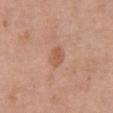The lesion was tiled from a total-body skin photograph and was not biopsied.
Measured at roughly 3 mm in maximum diameter.
The subject is a female about 60 years old.
Cropped from a whole-body photographic skin survey; the tile spans about 15 mm.
Captured under white-light illumination.
The lesion is located on the abdomen.